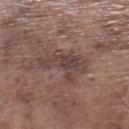<tbp_lesion>
<biopsy_status>not biopsied; imaged during a skin examination</biopsy_status>
<site>left lower leg</site>
<image>
  <source>total-body photography crop</source>
  <field_of_view_mm>15</field_of_view_mm>
</image>
<patient>
  <sex>male</sex>
  <age_approx>75</age_approx>
</patient>
</tbp_lesion>